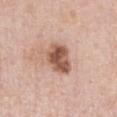Acquisition and patient details:
Automated image analysis of the tile measured a lesion color around L≈55 a*≈22 b*≈29 in CIELAB, a lesion–skin lightness drop of about 16, and a normalized border contrast of about 10.5. The analysis additionally found an automated nevus-likeness rating near 80 out of 100 and a detector confidence of about 100 out of 100 that the crop contains a lesion. About 4.5 mm across. The tile uses white-light illumination. The patient is a male aged 48–52. Cropped from a total-body skin-imaging series; the visible field is about 15 mm. On the front of the torso.